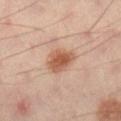<record>
  <site>leg</site>
  <automated_metrics>
    <eccentricity>0.7</eccentricity>
    <shape_asymmetry>0.25</shape_asymmetry>
    <cielab_L>55</cielab_L>
    <cielab_a>22</cielab_a>
    <cielab_b>31</cielab_b>
    <vs_skin_contrast_norm>8.5</vs_skin_contrast_norm>
  </automated_metrics>
  <image>
    <source>total-body photography crop</source>
    <field_of_view_mm>15</field_of_view_mm>
  </image>
  <lighting>cross-polarized</lighting>
  <patient>
    <sex>male</sex>
    <age_approx>40</age_approx>
  </patient>
</record>